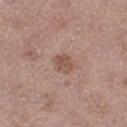| key | value |
|---|---|
| workup | imaged on a skin check; not biopsied |
| acquisition | ~15 mm tile from a whole-body skin photo |
| lighting | white-light illumination |
| lesion diameter | ~2.5 mm (longest diameter) |
| image-analysis metrics | a lesion area of about 3.5 mm² and an outline eccentricity of about 0.7 (0 = round, 1 = elongated); about 9 CIELAB-L* units darker than the surrounding skin and a normalized lesion–skin contrast near 6.5; border irregularity of about 3 on a 0–10 scale and a within-lesion color-variation index near 1.5/10 |
| subject | female, aged around 50 |
| anatomic site | the right thigh |